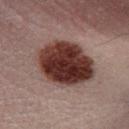biopsy_status: not biopsied; imaged during a skin examination
patient:
  sex: male
  age_approx: 50
image:
  source: total-body photography crop
  field_of_view_mm: 15
site: right lower leg
automated_metrics:
  area_mm2_approx: 31.0
  eccentricity: 0.65
  shape_asymmetry: 0.1
  cielab_L: 30
  cielab_a: 19
  cielab_b: 19
  vs_skin_darker_L: 18.0
  vs_skin_contrast_norm: 15.5
lesion_size:
  long_diameter_mm_approx: 7.5
lighting: cross-polarized